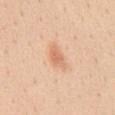Assessment:
This lesion was catalogued during total-body skin photography and was not selected for biopsy.
Context:
The lesion-visualizer software estimated a mean CIELAB color near L≈69 a*≈23 b*≈35, roughly 9 lightness units darker than nearby skin, and a normalized border contrast of about 5.5. And it measured a lesion-detection confidence of about 100/100. About 4 mm across. A lesion tile, about 15 mm wide, cut from a 3D total-body photograph. The tile uses white-light illumination. From the chest. A female subject approximately 45 years of age.